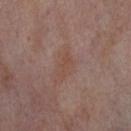<record>
  <biopsy_status>not biopsied; imaged during a skin examination</biopsy_status>
  <patient>
    <sex>female</sex>
    <age_approx>55</age_approx>
  </patient>
  <lesion_size>
    <long_diameter_mm_approx>3.0</long_diameter_mm_approx>
  </lesion_size>
  <automated_metrics>
    <eccentricity>0.95</eccentricity>
    <shape_asymmetry>0.45</shape_asymmetry>
    <color_variation_0_10>0.0</color_variation_0_10>
    <nevus_likeness_0_100>0</nevus_likeness_0_100>
    <lesion_detection_confidence_0_100>100</lesion_detection_confidence_0_100>
  </automated_metrics>
  <image>
    <source>total-body photography crop</source>
    <field_of_view_mm>15</field_of_view_mm>
  </image>
  <lighting>cross-polarized</lighting>
  <site>right thigh</site>
</record>